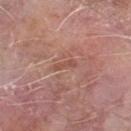The lesion was photographed on a routine skin check and not biopsied; there is no pathology result.
Cropped from a whole-body photographic skin survey; the tile spans about 15 mm.
Located on the left forearm.
A male subject, aged 63 to 67.